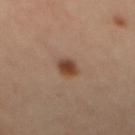A region of skin cropped from a whole-body photographic capture, roughly 15 mm wide. The lesion's longest dimension is about 3 mm. The lesion is on the mid back. The patient is a female about 35 years old. Imaged with cross-polarized lighting.